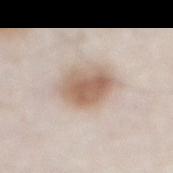{"biopsy_status": "not biopsied; imaged during a skin examination", "lesion_size": {"long_diameter_mm_approx": 5.0}, "patient": {"sex": "male", "age_approx": 80}, "site": "abdomen", "image": {"source": "total-body photography crop", "field_of_view_mm": 15}, "lighting": "white-light"}